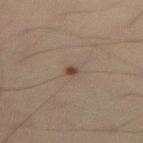Clinical impression:
Part of a total-body skin-imaging series; this lesion was reviewed on a skin check and was not flagged for biopsy.
Context:
The lesion is on the right thigh. Approximately 1.5 mm at its widest. A roughly 15 mm field-of-view crop from a total-body skin photograph. A male subject about 30 years old. An algorithmic analysis of the crop reported an outline eccentricity of about 0.55 (0 = round, 1 = elongated) and a symmetry-axis asymmetry near 0.35. The analysis additionally found a border-irregularity rating of about 2.5/10 and a peripheral color-asymmetry measure near 0.